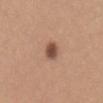notes = no biopsy performed (imaged during a skin exam) | subject = female, about 30 years old | site = the mid back | image = ~15 mm tile from a whole-body skin photo | tile lighting = white-light | size = about 2.5 mm.